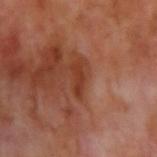{
  "site": "right upper arm",
  "lighting": "cross-polarized",
  "patient": {
    "sex": "male",
    "age_approx": 70
  },
  "image": {
    "source": "total-body photography crop",
    "field_of_view_mm": 15
  }
}